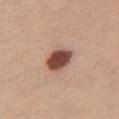biopsy_status: not biopsied; imaged during a skin examination
site: chest
automated_metrics:
  area_mm2_approx: 8.0
  eccentricity: 0.7
image:
  source: total-body photography crop
  field_of_view_mm: 15
patient:
  sex: female
  age_approx: 25
lighting: white-light
lesion_size:
  long_diameter_mm_approx: 3.5